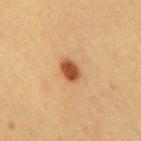<record>
<biopsy_status>not biopsied; imaged during a skin examination</biopsy_status>
<patient>
  <sex>female</sex>
  <age_approx>20</age_approx>
</patient>
<lighting>cross-polarized</lighting>
<automated_metrics>
  <area_mm2_approx>5.0</area_mm2_approx>
  <eccentricity>0.7</eccentricity>
  <shape_asymmetry>0.2</shape_asymmetry>
  <border_irregularity_0_10>2.0</border_irregularity_0_10>
  <color_variation_0_10>3.5</color_variation_0_10>
  <peripheral_color_asymmetry>1.0</peripheral_color_asymmetry>
</automated_metrics>
<image>
  <source>total-body photography crop</source>
  <field_of_view_mm>15</field_of_view_mm>
</image>
<site>back</site>
<lesion_size>
  <long_diameter_mm_approx>3.0</long_diameter_mm_approx>
</lesion_size>
</record>